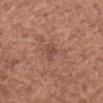| feature | finding |
|---|---|
| workup | total-body-photography surveillance lesion; no biopsy |
| lesion size | about 2.5 mm |
| automated metrics | a border-irregularity index near 3.5/10, a color-variation rating of about 1/10, and a peripheral color-asymmetry measure near 0 |
| image source | total-body-photography crop, ~15 mm field of view |
| body site | the left forearm |
| patient | female, aged 48 to 52 |